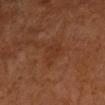workup = no biopsy performed (imaged during a skin exam)
acquisition = total-body-photography crop, ~15 mm field of view
subject = female, about 65 years old
location = the left forearm